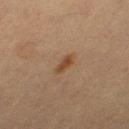Findings:
• notes: catalogued during a skin exam; not biopsied
• patient: female, approximately 55 years of age
• automated lesion analysis: a footprint of about 4 mm², an outline eccentricity of about 0.9 (0 = round, 1 = elongated), and a shape-asymmetry score of about 0.25 (0 = symmetric); a lesion color around L≈37 a*≈16 b*≈28 in CIELAB, a lesion–skin lightness drop of about 7, and a normalized border contrast of about 7.5; a border-irregularity rating of about 2.5/10 and a within-lesion color-variation index near 1.5/10; a lesion-detection confidence of about 100/100
• imaging modality: ~15 mm crop, total-body skin-cancer survey
• anatomic site: the right thigh
• lighting: cross-polarized illumination
• lesion diameter: ≈3 mm